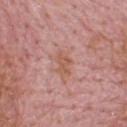The lesion was photographed on a routine skin check and not biopsied; there is no pathology result.
About 3 mm across.
Imaged with white-light lighting.
A male patient, aged 63 to 67.
A lesion tile, about 15 mm wide, cut from a 3D total-body photograph.
The lesion is on the upper back.